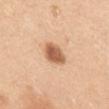Assessment: Part of a total-body skin-imaging series; this lesion was reviewed on a skin check and was not flagged for biopsy. Context: Cropped from a whole-body photographic skin survey; the tile spans about 15 mm. A female subject approximately 30 years of age. Located on the mid back.